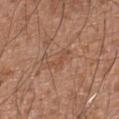Background:
The tile uses white-light illumination. Longest diameter approximately 3.5 mm. A male patient, aged approximately 75. This image is a 15 mm lesion crop taken from a total-body photograph. The lesion is located on the abdomen. Automated tile analysis of the lesion measured a lesion area of about 2.5 mm², an eccentricity of roughly 0.95, and a shape-asymmetry score of about 0.5 (0 = symmetric).